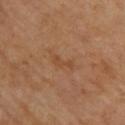Q: Is there a histopathology result?
A: no biopsy performed (imaged during a skin exam)
Q: Where on the body is the lesion?
A: the upper back
Q: Automated lesion metrics?
A: a mean CIELAB color near L≈47 a*≈22 b*≈35 and about 6 CIELAB-L* units darker than the surrounding skin; a border-irregularity rating of about 6/10 and a peripheral color-asymmetry measure near 0
Q: How was this image acquired?
A: 15 mm crop, total-body photography
Q: Lesion size?
A: about 2.5 mm